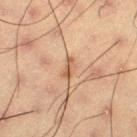follow-up: imaged on a skin check; not biopsied | anatomic site: the right thigh | image source: ~15 mm crop, total-body skin-cancer survey | subject: male, in their mid- to late 50s.